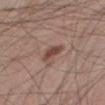Part of a total-body skin-imaging series; this lesion was reviewed on a skin check and was not flagged for biopsy.
Cropped from a whole-body photographic skin survey; the tile spans about 15 mm.
Longest diameter approximately 3.5 mm.
A male subject aged around 45.
On the leg.